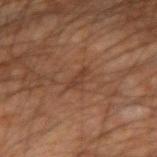Findings:
* notes: total-body-photography surveillance lesion; no biopsy
* subject: male, aged 63–67
* image source: 15 mm crop, total-body photography
* automated metrics: an area of roughly 2.5 mm², a shape eccentricity near 0.95, and a shape-asymmetry score of about 0.35 (0 = symmetric); an average lesion color of about L≈32 a*≈18 b*≈25 (CIELAB), a lesion–skin lightness drop of about 6, and a lesion-to-skin contrast of about 5.5 (normalized; higher = more distinct); a within-lesion color-variation index near 0/10 and a peripheral color-asymmetry measure near 0; a classifier nevus-likeness of about 0/100
* site: the arm